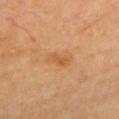Notes:
- biopsy status: total-body-photography surveillance lesion; no biopsy
- image: 15 mm crop, total-body photography
- patient: female, approximately 70 years of age
- body site: the chest